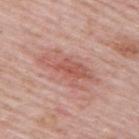No biopsy was performed on this lesion — it was imaged during a full skin examination and was not determined to be concerning.
From the back.
About 6 mm across.
A male subject approximately 65 years of age.
Captured under white-light illumination.
Cropped from a whole-body photographic skin survey; the tile spans about 15 mm.